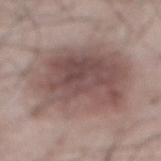Q: Was a biopsy performed?
A: total-body-photography surveillance lesion; no biopsy
Q: Automated lesion metrics?
A: an outline eccentricity of about 0.5 (0 = round, 1 = elongated) and a shape-asymmetry score of about 0.15 (0 = symmetric); a color-variation rating of about 7/10 and a peripheral color-asymmetry measure near 2.5; a classifier nevus-likeness of about 80/100 and a detector confidence of about 100 out of 100 that the crop contains a lesion
Q: What kind of image is this?
A: total-body-photography crop, ~15 mm field of view
Q: Lesion location?
A: the abdomen
Q: Illumination type?
A: white-light
Q: Patient demographics?
A: male, in their mid- to late 50s
Q: What is the lesion's diameter?
A: ~10 mm (longest diameter)